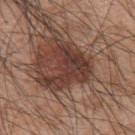Notes:
* workup · total-body-photography surveillance lesion; no biopsy
* size · ≈5 mm
* subject · male, about 45 years old
* tile lighting · white-light
* anatomic site · the upper back
* automated lesion analysis · an outline eccentricity of about 0.55 (0 = round, 1 = elongated) and a symmetry-axis asymmetry near 0.65; a lesion color around L≈37 a*≈20 b*≈24 in CIELAB and roughly 12 lightness units darker than nearby skin; an automated nevus-likeness rating near 75 out of 100 and lesion-presence confidence of about 100/100
* image · total-body-photography crop, ~15 mm field of view